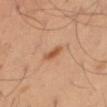Recorded during total-body skin imaging; not selected for excision or biopsy. Captured under cross-polarized illumination. A male patient, about 50 years old. The lesion-visualizer software estimated a lesion area of about 3 mm², an outline eccentricity of about 0.9 (0 = round, 1 = elongated), and two-axis asymmetry of about 0.35. And it measured a mean CIELAB color near L≈53 a*≈24 b*≈36, roughly 11 lightness units darker than nearby skin, and a normalized lesion–skin contrast near 7.5. The software also gave a border-irregularity rating of about 3.5/10, a color-variation rating of about 0/10, and radial color variation of about 0. And it measured a classifier nevus-likeness of about 95/100 and lesion-presence confidence of about 100/100. Measured at roughly 3 mm in maximum diameter. Located on the right thigh. A roughly 15 mm field-of-view crop from a total-body skin photograph.